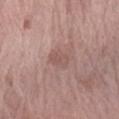Impression:
The lesion was photographed on a routine skin check and not biopsied; there is no pathology result.
Clinical summary:
Captured under white-light illumination. A female subject approximately 75 years of age. A roughly 15 mm field-of-view crop from a total-body skin photograph. Longest diameter approximately 3 mm. From the left forearm. Automated tile analysis of the lesion measured a footprint of about 4 mm², a shape eccentricity near 0.75, and a shape-asymmetry score of about 0.2 (0 = symmetric). The software also gave a nevus-likeness score of about 0/100 and lesion-presence confidence of about 100/100.